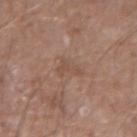Q: Was this lesion biopsied?
A: total-body-photography surveillance lesion; no biopsy
Q: What is the anatomic site?
A: the arm
Q: What did automated image analysis measure?
A: a lesion area of about 3.5 mm² and two-axis asymmetry of about 0.65; an automated nevus-likeness rating near 0 out of 100 and a lesion-detection confidence of about 100/100
Q: What is the imaging modality?
A: ~15 mm crop, total-body skin-cancer survey
Q: How large is the lesion?
A: ≈3 mm
Q: What are the patient's age and sex?
A: male, aged 73–77
Q: What lighting was used for the tile?
A: white-light illumination